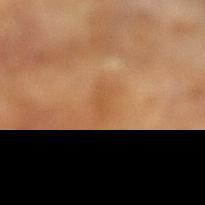A region of skin cropped from a whole-body photographic capture, roughly 15 mm wide. The subject is a male approximately 65 years of age. Automated tile analysis of the lesion measured a normalized lesion–skin contrast near 4.5. The software also gave an automated nevus-likeness rating near 0 out of 100 and a detector confidence of about 100 out of 100 that the crop contains a lesion.